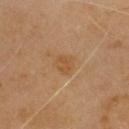Part of a total-body skin-imaging series; this lesion was reviewed on a skin check and was not flagged for biopsy. A lesion tile, about 15 mm wide, cut from a 3D total-body photograph. A male patient aged 58 to 62. Automated image analysis of the tile measured a lesion area of about 4 mm², an eccentricity of roughly 0.7, and a symmetry-axis asymmetry near 0.3. This is a cross-polarized tile. The lesion is on the head or neck.